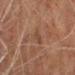<case>
  <biopsy_status>not biopsied; imaged during a skin examination</biopsy_status>
  <site>right lower leg</site>
  <image>
    <source>total-body photography crop</source>
    <field_of_view_mm>15</field_of_view_mm>
  </image>
  <patient>
    <sex>male</sex>
    <age_approx>70</age_approx>
  </patient>
</case>